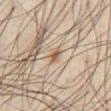Impression: This lesion was catalogued during total-body skin photography and was not selected for biopsy. Image and clinical context: Longest diameter approximately 2 mm. On the abdomen. This is a cross-polarized tile. A close-up tile cropped from a whole-body skin photograph, about 15 mm across. The lesion-visualizer software estimated a lesion area of about 2 mm² and an outline eccentricity of about 0.85 (0 = round, 1 = elongated). The analysis additionally found an average lesion color of about L≈55 a*≈15 b*≈30 (CIELAB) and a normalized lesion–skin contrast near 8.5. The software also gave an automated nevus-likeness rating near 85 out of 100 and lesion-presence confidence of about 80/100. A male patient, in their mid- to late 40s.